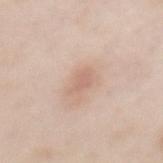Q: Is there a histopathology result?
A: catalogued during a skin exam; not biopsied
Q: What is the imaging modality?
A: 15 mm crop, total-body photography
Q: What are the patient's age and sex?
A: female, in their 50s
Q: What is the lesion's diameter?
A: ≈3 mm
Q: What did automated image analysis measure?
A: an area of roughly 3 mm², a shape eccentricity near 0.85, and two-axis asymmetry of about 0.3; border irregularity of about 3.5 on a 0–10 scale, internal color variation of about 1.5 on a 0–10 scale, and a peripheral color-asymmetry measure near 0.5; a classifier nevus-likeness of about 10/100
Q: What is the anatomic site?
A: the mid back